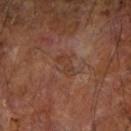No biopsy was performed on this lesion — it was imaged during a full skin examination and was not determined to be concerning.
A male subject in their mid-60s.
A 15 mm close-up extracted from a 3D total-body photography capture.
On the right forearm.